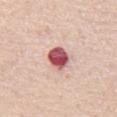Findings:
– subject — male, approximately 40 years of age
– body site — the chest
– size — ≈3.5 mm
– image — total-body-photography crop, ~15 mm field of view
– lighting — white-light